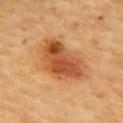Case summary:
- lighting — cross-polarized illumination
- lesion size — ≈6.5 mm
- acquisition — 15 mm crop, total-body photography
- location — the upper back
- image-analysis metrics — an average lesion color of about L≈46 a*≈25 b*≈37 (CIELAB), about 12 CIELAB-L* units darker than the surrounding skin, and a normalized lesion–skin contrast near 9; border irregularity of about 3 on a 0–10 scale, a within-lesion color-variation index near 7/10, and a peripheral color-asymmetry measure near 2; an automated nevus-likeness rating near 100 out of 100
- subject — female, aged 58 to 62